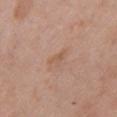Captured during whole-body skin photography for melanoma surveillance; the lesion was not biopsied. Located on the front of the torso. Imaged with white-light lighting. Cropped from a whole-body photographic skin survey; the tile spans about 15 mm. The subject is a female approximately 60 years of age.